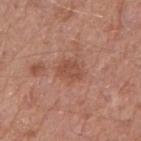Clinical impression:
This lesion was catalogued during total-body skin photography and was not selected for biopsy.